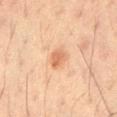  patient:
    sex: male
    age_approx: 35
  site: leg
  image:
    source: total-body photography crop
    field_of_view_mm: 15